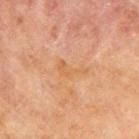Assessment: Imaged during a routine full-body skin examination; the lesion was not biopsied and no histopathology is available. Image and clinical context: The tile uses cross-polarized illumination. The lesion is on the upper back. Measured at roughly 4 mm in maximum diameter. A male subject, roughly 70 years of age. A 15 mm close-up tile from a total-body photography series done for melanoma screening.